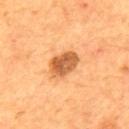Q: What is the anatomic site?
A: the mid back
Q: What is the lesion's diameter?
A: ≈4 mm
Q: What lighting was used for the tile?
A: cross-polarized illumination
Q: What is the imaging modality?
A: 15 mm crop, total-body photography
Q: Patient demographics?
A: male, approximately 55 years of age